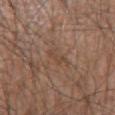Notes:
* biopsy status — catalogued during a skin exam; not biopsied
* automated metrics — a mean CIELAB color near L≈45 a*≈17 b*≈27 and a normalized border contrast of about 4.5; border irregularity of about 5.5 on a 0–10 scale, internal color variation of about 0 on a 0–10 scale, and a peripheral color-asymmetry measure near 0
* acquisition — ~15 mm crop, total-body skin-cancer survey
* lighting — white-light
* body site — the right upper arm
* subject — male, about 60 years old
* diameter — ~3 mm (longest diameter)